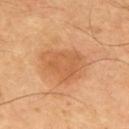Imaged during a routine full-body skin examination; the lesion was not biopsied and no histopathology is available.
A 15 mm close-up tile from a total-body photography series done for melanoma screening.
Captured under cross-polarized illumination.
Automated tile analysis of the lesion measured a footprint of about 16 mm² and an eccentricity of roughly 0.7. It also reported a lesion color around L≈58 a*≈24 b*≈40 in CIELAB and about 8 CIELAB-L* units darker than the surrounding skin. The analysis additionally found a nevus-likeness score of about 35/100 and a lesion-detection confidence of about 100/100.
From the back.
Longest diameter approximately 5.5 mm.
A male subject roughly 55 years of age.